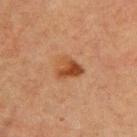Imaged during a routine full-body skin examination; the lesion was not biopsied and no histopathology is available.
On the upper back.
This image is a 15 mm lesion crop taken from a total-body photograph.
The subject is a male about 60 years old.